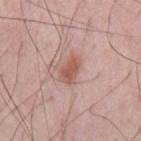{
  "biopsy_status": "not biopsied; imaged during a skin examination",
  "image": {
    "source": "total-body photography crop",
    "field_of_view_mm": 15
  },
  "site": "mid back",
  "patient": {
    "sex": "male",
    "age_approx": 50
  },
  "lighting": "white-light",
  "lesion_size": {
    "long_diameter_mm_approx": 3.0
  }
}